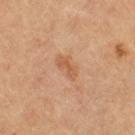patient = female, aged 58–62; body site = the right thigh; acquisition = 15 mm crop, total-body photography.